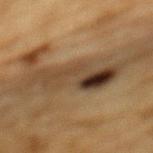Located on the mid back. The patient is a male approximately 85 years of age or older. Automated image analysis of the tile measured a lesion area of about 19 mm², an eccentricity of roughly 0.95, and two-axis asymmetry of about 0.4. The analysis additionally found a lesion–skin lightness drop of about 10 and a lesion-to-skin contrast of about 10 (normalized; higher = more distinct). The lesion's longest dimension is about 8 mm. A 15 mm close-up extracted from a 3D total-body photography capture.